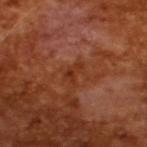Case summary:
* follow-up — imaged on a skin check; not biopsied
* illumination — cross-polarized
* lesion diameter — ≈2.5 mm
* acquisition — total-body-photography crop, ~15 mm field of view
* patient — male, aged 63–67